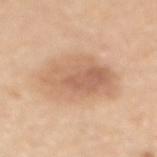Captured during whole-body skin photography for melanoma surveillance; the lesion was not biopsied.
About 7 mm across.
The lesion is located on the mid back.
A close-up tile cropped from a whole-body skin photograph, about 15 mm across.
A female patient aged 43 to 47.
Automated image analysis of the tile measured a shape-asymmetry score of about 0.2 (0 = symmetric). It also reported an automated nevus-likeness rating near 30 out of 100 and a detector confidence of about 100 out of 100 that the crop contains a lesion.
Captured under white-light illumination.